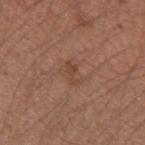Assessment:
The lesion was photographed on a routine skin check and not biopsied; there is no pathology result.